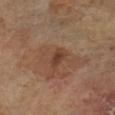{
  "biopsy_status": "not biopsied; imaged during a skin examination",
  "site": "leg",
  "lesion_size": {
    "long_diameter_mm_approx": 2.5
  },
  "lighting": "cross-polarized",
  "patient": {
    "sex": "female",
    "age_approx": 70
  },
  "automated_metrics": {
    "cielab_L": 37,
    "cielab_a": 20,
    "cielab_b": 27,
    "border_irregularity_0_10": 2.5,
    "color_variation_0_10": 2.0,
    "peripheral_color_asymmetry": 0.5
  },
  "image": {
    "source": "total-body photography crop",
    "field_of_view_mm": 15
  }
}